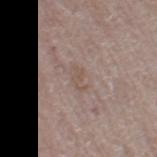Q: Is there a histopathology result?
A: total-body-photography surveillance lesion; no biopsy
Q: What did automated image analysis measure?
A: a footprint of about 3.5 mm², a shape eccentricity near 0.85, and two-axis asymmetry of about 0.4; roughly 6 lightness units darker than nearby skin and a normalized lesion–skin contrast near 5; an automated nevus-likeness rating near 0 out of 100 and a detector confidence of about 100 out of 100 that the crop contains a lesion
Q: Lesion size?
A: about 3 mm
Q: What are the patient's age and sex?
A: female, aged 63 to 67
Q: Where on the body is the lesion?
A: the right thigh
Q: What is the imaging modality?
A: ~15 mm crop, total-body skin-cancer survey
Q: What lighting was used for the tile?
A: white-light illumination